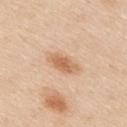| feature | finding |
|---|---|
| notes | total-body-photography surveillance lesion; no biopsy |
| lighting | white-light illumination |
| automated lesion analysis | a lesion area of about 6 mm² and two-axis asymmetry of about 0.3; a border-irregularity rating of about 3/10 and peripheral color asymmetry of about 1 |
| diameter | ~4 mm (longest diameter) |
| subject | male, approximately 35 years of age |
| location | the upper back |
| image source | ~15 mm tile from a whole-body skin photo |